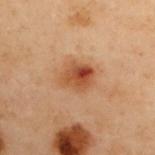biopsy_status: not biopsied; imaged during a skin examination
image:
  source: total-body photography crop
  field_of_view_mm: 15
site: upper back
patient:
  sex: female
  age_approx: 60